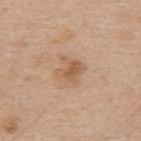Located on the upper back. A male patient, aged 68 to 72. A 15 mm close-up extracted from a 3D total-body photography capture.